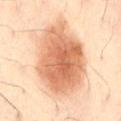Notes:
• biopsy status: total-body-photography surveillance lesion; no biopsy